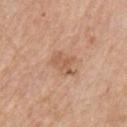Case summary:
- workup — no biopsy performed (imaged during a skin exam)
- body site — the right upper arm
- acquisition — total-body-photography crop, ~15 mm field of view
- diameter — about 3.5 mm
- patient — male, about 65 years old